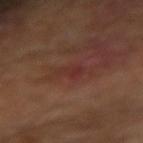Part of a total-body skin-imaging series; this lesion was reviewed on a skin check and was not flagged for biopsy. The tile uses cross-polarized illumination. The lesion is located on the right forearm. A male patient, aged around 65. Automated image analysis of the tile measured an area of roughly 3.5 mm², an outline eccentricity of about 0.8 (0 = round, 1 = elongated), and a shape-asymmetry score of about 0.35 (0 = symmetric). The software also gave a within-lesion color-variation index near 1/10 and radial color variation of about 0.5. The analysis additionally found a classifier nevus-likeness of about 0/100 and a lesion-detection confidence of about 100/100. A roughly 15 mm field-of-view crop from a total-body skin photograph. The lesion's longest dimension is about 2.5 mm.